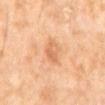Q: Was this lesion biopsied?
A: no biopsy performed (imaged during a skin exam)
Q: Lesion size?
A: ~3 mm (longest diameter)
Q: Where on the body is the lesion?
A: the front of the torso
Q: Patient demographics?
A: male, aged around 65
Q: What is the imaging modality?
A: total-body-photography crop, ~15 mm field of view